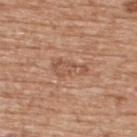| key | value |
|---|---|
| biopsy status | no biopsy performed (imaged during a skin exam) |
| image | ~15 mm crop, total-body skin-cancer survey |
| subject | male, about 60 years old |
| location | the upper back |
| lighting | white-light |
| image-analysis metrics | a footprint of about 7 mm² and two-axis asymmetry of about 0.4; a lesion color around L≈54 a*≈22 b*≈30 in CIELAB and a lesion–skin lightness drop of about 7; an automated nevus-likeness rating near 0 out of 100 and a detector confidence of about 100 out of 100 that the crop contains a lesion |
| diameter | ≈4.5 mm |